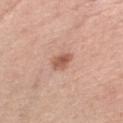Assessment: Part of a total-body skin-imaging series; this lesion was reviewed on a skin check and was not flagged for biopsy. Context: Imaged with white-light lighting. Automated image analysis of the tile measured a nevus-likeness score of about 75/100 and lesion-presence confidence of about 100/100. A 15 mm close-up tile from a total-body photography series done for melanoma screening. From the left forearm. The patient is a female approximately 50 years of age.